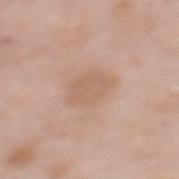{"patient": {"sex": "female", "age_approx": 50}, "lesion_size": {"long_diameter_mm_approx": 4.5}, "image": {"source": "total-body photography crop", "field_of_view_mm": 15}, "lighting": "white-light", "site": "upper back"}